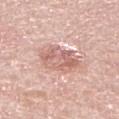Imaged during a routine full-body skin examination; the lesion was not biopsied and no histopathology is available.
Located on the right lower leg.
This image is a 15 mm lesion crop taken from a total-body photograph.
A male subject, in their 70s.